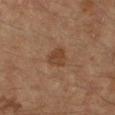This lesion was catalogued during total-body skin photography and was not selected for biopsy.
Measured at roughly 3 mm in maximum diameter.
Automated tile analysis of the lesion measured a lesion area of about 5 mm² and a shape eccentricity near 0.8. The software also gave an average lesion color of about L≈43 a*≈20 b*≈32 (CIELAB), roughly 8 lightness units darker than nearby skin, and a normalized lesion–skin contrast near 7. It also reported border irregularity of about 2.5 on a 0–10 scale, a within-lesion color-variation index near 2.5/10, and a peripheral color-asymmetry measure near 1. The analysis additionally found a lesion-detection confidence of about 100/100.
A roughly 15 mm field-of-view crop from a total-body skin photograph.
The lesion is located on the right forearm.
A female patient in their 60s.